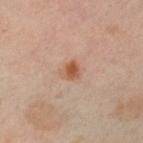notes: catalogued during a skin exam; not biopsied
acquisition: 15 mm crop, total-body photography
TBP lesion metrics: a lesion color around L≈56 a*≈24 b*≈34 in CIELAB; a detector confidence of about 100 out of 100 that the crop contains a lesion
size: ≈2 mm
site: the left lower leg
tile lighting: cross-polarized illumination
subject: female, aged around 50